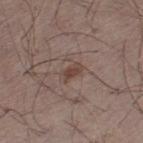Q: Is there a histopathology result?
A: total-body-photography surveillance lesion; no biopsy
Q: What kind of image is this?
A: ~15 mm tile from a whole-body skin photo
Q: Who is the patient?
A: male, roughly 50 years of age
Q: Lesion size?
A: ≈2.5 mm
Q: How was the tile lit?
A: white-light
Q: Where on the body is the lesion?
A: the left thigh